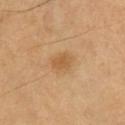Q: Was a biopsy performed?
A: total-body-photography surveillance lesion; no biopsy